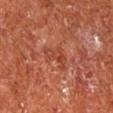Q: Is there a histopathology result?
A: total-body-photography surveillance lesion; no biopsy
Q: Where on the body is the lesion?
A: the right lower leg
Q: How was this image acquired?
A: ~15 mm tile from a whole-body skin photo
Q: Who is the patient?
A: male, aged approximately 65